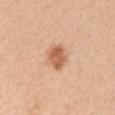This lesion was catalogued during total-body skin photography and was not selected for biopsy. Imaged with white-light lighting. Automated tile analysis of the lesion measured an area of roughly 7 mm² and a symmetry-axis asymmetry near 0.2. The patient is a female aged approximately 40. A roughly 15 mm field-of-view crop from a total-body skin photograph. The lesion is located on the right upper arm.